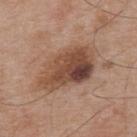image source — ~15 mm crop, total-body skin-cancer survey | lesion diameter — about 7 mm | illumination — white-light illumination | subject — male, in their mid-50s | site — the upper back.